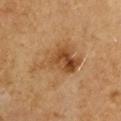<case>
<biopsy_status>not biopsied; imaged during a skin examination</biopsy_status>
<lighting>cross-polarized</lighting>
<image>
  <source>total-body photography crop</source>
  <field_of_view_mm>15</field_of_view_mm>
</image>
<site>right upper arm</site>
<automated_metrics>
  <cielab_L>45</cielab_L>
  <cielab_a>19</cielab_a>
  <cielab_b>35</cielab_b>
  <vs_skin_darker_L>9.0</vs_skin_darker_L>
  <border_irregularity_0_10>6.0</border_irregularity_0_10>
  <color_variation_0_10>8.5</color_variation_0_10>
  <peripheral_color_asymmetry>3.5</peripheral_color_asymmetry>
</automated_metrics>
<patient>
  <sex>male</sex>
  <age_approx>60</age_approx>
</patient>
<lesion_size>
  <long_diameter_mm_approx>7.0</long_diameter_mm_approx>
</lesion_size>
</case>